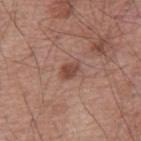On the mid back.
Imaged with white-light lighting.
The recorded lesion diameter is about 2.5 mm.
A male subject, aged approximately 55.
Automated tile analysis of the lesion measured a lesion area of about 3.5 mm², a shape eccentricity near 0.75, and two-axis asymmetry of about 0.3. The software also gave a border-irregularity index near 3/10, a color-variation rating of about 2/10, and radial color variation of about 0.5. The analysis additionally found lesion-presence confidence of about 100/100.
A roughly 15 mm field-of-view crop from a total-body skin photograph.